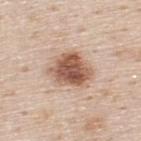workup=imaged on a skin check; not biopsied
subject=male, in their mid-40s
acquisition=~15 mm crop, total-body skin-cancer survey
site=the upper back
automated lesion analysis=a nevus-likeness score of about 95/100 and lesion-presence confidence of about 100/100
size=~5 mm (longest diameter)